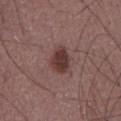biopsy status: catalogued during a skin exam; not biopsied | subject: male, roughly 60 years of age | size: about 3.5 mm | imaging modality: ~15 mm crop, total-body skin-cancer survey | lighting: white-light | body site: the abdomen.